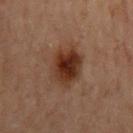Q: Is there a histopathology result?
A: no biopsy performed (imaged during a skin exam)
Q: How was this image acquired?
A: ~15 mm crop, total-body skin-cancer survey
Q: Lesion location?
A: the mid back
Q: Who is the patient?
A: male, approximately 70 years of age